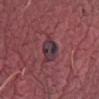Case summary:
- workup: no biopsy performed (imaged during a skin exam)
- patient: male, about 70 years old
- lesion diameter: ~5 mm (longest diameter)
- tile lighting: white-light
- body site: the left thigh
- TBP lesion metrics: a footprint of about 8 mm², a shape eccentricity near 0.85, and two-axis asymmetry of about 0.35; border irregularity of about 4 on a 0–10 scale, a color-variation rating of about 6/10, and radial color variation of about 2
- image source: ~15 mm tile from a whole-body skin photo